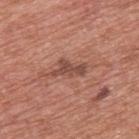notes: no biopsy performed (imaged during a skin exam) | illumination: white-light illumination | subject: male, aged approximately 70 | acquisition: total-body-photography crop, ~15 mm field of view | automated metrics: a normalized lesion–skin contrast near 7.5; a classifier nevus-likeness of about 0/100 and a lesion-detection confidence of about 95/100 | anatomic site: the upper back.